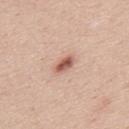follow-up = catalogued during a skin exam; not biopsied | anatomic site = the upper back | automated lesion analysis = an eccentricity of roughly 0.85 and a symmetry-axis asymmetry near 0.2 | image source = total-body-photography crop, ~15 mm field of view | lesion diameter = ≈3 mm | tile lighting = white-light illumination | subject = male, in their mid- to late 40s.